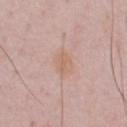Q: Was a biopsy performed?
A: imaged on a skin check; not biopsied
Q: How was this image acquired?
A: ~15 mm tile from a whole-body skin photo
Q: What lighting was used for the tile?
A: white-light illumination
Q: Where on the body is the lesion?
A: the chest
Q: Who is the patient?
A: male, in their 50s